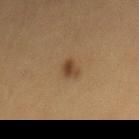| field | value |
|---|---|
| image source | ~15 mm crop, total-body skin-cancer survey |
| patient | female, approximately 40 years of age |
| location | the mid back |
| diameter | ~2.5 mm (longest diameter) |
| automated metrics | border irregularity of about 3 on a 0–10 scale and internal color variation of about 2 on a 0–10 scale; a classifier nevus-likeness of about 95/100 and a detector confidence of about 100 out of 100 that the crop contains a lesion |
| illumination | cross-polarized |